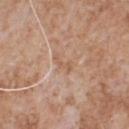{
  "biopsy_status": "not biopsied; imaged during a skin examination",
  "image": {
    "source": "total-body photography crop",
    "field_of_view_mm": 15
  },
  "site": "chest",
  "patient": {
    "sex": "male",
    "age_approx": 65
  }
}